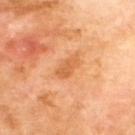Impression:
Imaged during a routine full-body skin examination; the lesion was not biopsied and no histopathology is available.
Acquisition and patient details:
The tile uses cross-polarized illumination. Located on the upper back. A close-up tile cropped from a whole-body skin photograph, about 15 mm across. The lesion-visualizer software estimated a lesion area of about 5 mm², an outline eccentricity of about 0.85 (0 = round, 1 = elongated), and two-axis asymmetry of about 0.25. The analysis additionally found border irregularity of about 2.5 on a 0–10 scale, internal color variation of about 2.5 on a 0–10 scale, and radial color variation of about 1. The analysis additionally found an automated nevus-likeness rating near 5 out of 100. The patient is a male about 70 years old.